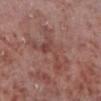{"biopsy_status": "not biopsied; imaged during a skin examination", "lighting": "white-light", "lesion_size": {"long_diameter_mm_approx": 6.5}, "patient": {"sex": "male", "age_approx": 55}, "automated_metrics": {"cielab_L": 46, "cielab_a": 22, "cielab_b": 23, "vs_skin_darker_L": 7.0, "vs_skin_contrast_norm": 5.0, "border_irregularity_0_10": 9.0, "color_variation_0_10": 5.0, "peripheral_color_asymmetry": 1.5}, "image": {"source": "total-body photography crop", "field_of_view_mm": 15}, "site": "left lower leg"}